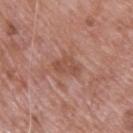{
  "biopsy_status": "not biopsied; imaged during a skin examination",
  "automated_metrics": {
    "area_mm2_approx": 5.5,
    "eccentricity": 0.7,
    "cielab_L": 50,
    "cielab_a": 23,
    "cielab_b": 28,
    "vs_skin_darker_L": 8.0,
    "lesion_detection_confidence_0_100": 100
  },
  "site": "back",
  "patient": {
    "sex": "male",
    "age_approx": 70
  },
  "lighting": "white-light",
  "lesion_size": {
    "long_diameter_mm_approx": 3.5
  },
  "image": {
    "source": "total-body photography crop",
    "field_of_view_mm": 15
  }
}